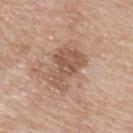notes — no biopsy performed (imaged during a skin exam) | site — the upper back | patient — male, aged around 65 | diameter — ~5 mm (longest diameter) | lighting — white-light | image source — total-body-photography crop, ~15 mm field of view.